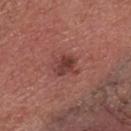No biopsy was performed on this lesion — it was imaged during a full skin examination and was not determined to be concerning. An algorithmic analysis of the crop reported an area of roughly 6 mm², an eccentricity of roughly 0.5, and a symmetry-axis asymmetry near 0.2. The software also gave roughly 10 lightness units darker than nearby skin and a lesion-to-skin contrast of about 8 (normalized; higher = more distinct). The software also gave border irregularity of about 2.5 on a 0–10 scale and radial color variation of about 2. It also reported a nevus-likeness score of about 75/100. About 3 mm across. Located on the head or neck. A region of skin cropped from a whole-body photographic capture, roughly 15 mm wide. A male subject, in their mid-70s. The tile uses white-light illumination.